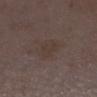Clinical impression:
No biopsy was performed on this lesion — it was imaged during a full skin examination and was not determined to be concerning.
Context:
A region of skin cropped from a whole-body photographic capture, roughly 15 mm wide. Captured under white-light illumination. A female patient, about 30 years old. From the right lower leg.